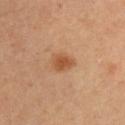Impression:
No biopsy was performed on this lesion — it was imaged during a full skin examination and was not determined to be concerning.
Image and clinical context:
A male subject, aged 33 to 37. A close-up tile cropped from a whole-body skin photograph, about 15 mm across. From the front of the torso.